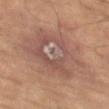notes: imaged on a skin check; not biopsied | image-analysis metrics: a lesion area of about 55 mm², an outline eccentricity of about 0.7 (0 = round, 1 = elongated), and a shape-asymmetry score of about 0.3 (0 = symmetric); a lesion color around L≈51 a*≈18 b*≈25 in CIELAB, a lesion–skin lightness drop of about 7, and a lesion-to-skin contrast of about 6 (normalized; higher = more distinct); a nevus-likeness score of about 0/100 and lesion-presence confidence of about 100/100 | size: ~11 mm (longest diameter) | patient: male, about 60 years old | illumination: cross-polarized illumination | imaging modality: ~15 mm tile from a whole-body skin photo | location: the left thigh.